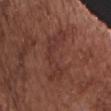The lesion was photographed on a routine skin check and not biopsied; there is no pathology result.
Cropped from a total-body skin-imaging series; the visible field is about 15 mm.
A male subject, in their mid- to late 70s.
The lesion is on the chest.